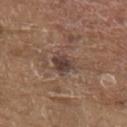Clinical impression: Captured during whole-body skin photography for melanoma surveillance; the lesion was not biopsied. Clinical summary: Measured at roughly 3.5 mm in maximum diameter. A roughly 15 mm field-of-view crop from a total-body skin photograph. The lesion is on the upper back. A male subject aged 78 to 82. This is a white-light tile.